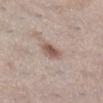Recorded during total-body skin imaging; not selected for excision or biopsy. About 2.5 mm across. A 15 mm close-up tile from a total-body photography series done for melanoma screening. The total-body-photography lesion software estimated an area of roughly 4.5 mm², an eccentricity of roughly 0.5, and two-axis asymmetry of about 0.25. The analysis additionally found a border-irregularity index near 2/10, internal color variation of about 3.5 on a 0–10 scale, and peripheral color asymmetry of about 1. It also reported a classifier nevus-likeness of about 90/100. Imaged with white-light lighting. From the right lower leg. A female patient aged around 40.